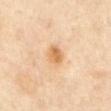{"biopsy_status": "not biopsied; imaged during a skin examination", "lighting": "cross-polarized", "site": "abdomen", "lesion_size": {"long_diameter_mm_approx": 3.0}, "automated_metrics": {"eccentricity": 0.65, "shape_asymmetry": 0.2, "cielab_L": 67, "cielab_a": 19, "cielab_b": 40, "vs_skin_contrast_norm": 7.5, "border_irregularity_0_10": 2.0, "color_variation_0_10": 3.5, "peripheral_color_asymmetry": 1.0}, "image": {"source": "total-body photography crop", "field_of_view_mm": 15}, "patient": {"sex": "female", "age_approx": 50}}